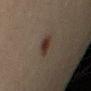This lesion was catalogued during total-body skin photography and was not selected for biopsy. The patient is a male aged 38–42. Located on the right upper arm. This image is a 15 mm lesion crop taken from a total-body photograph. The recorded lesion diameter is about 2.5 mm. The tile uses cross-polarized illumination.